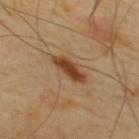{"biopsy_status": "not biopsied; imaged during a skin examination", "lesion_size": {"long_diameter_mm_approx": 4.5}, "site": "upper back", "patient": {"sex": "male", "age_approx": 65}, "image": {"source": "total-body photography crop", "field_of_view_mm": 15}, "automated_metrics": {"cielab_L": 42, "cielab_a": 21, "cielab_b": 35, "vs_skin_darker_L": 13.0, "vs_skin_contrast_norm": 10.5, "lesion_detection_confidence_0_100": 100}}